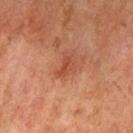The lesion was tiled from a total-body skin photograph and was not biopsied.
A female patient, approximately 60 years of age.
The lesion is on the left upper arm.
A 15 mm close-up tile from a total-body photography series done for melanoma screening.